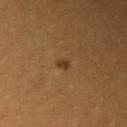Imaged during a routine full-body skin examination; the lesion was not biopsied and no histopathology is available.
The recorded lesion diameter is about 2 mm.
A 15 mm close-up extracted from a 3D total-body photography capture.
A female patient aged around 30.
Captured under cross-polarized illumination.
An algorithmic analysis of the crop reported a mean CIELAB color near L≈34 a*≈17 b*≈32, roughly 7 lightness units darker than nearby skin, and a lesion-to-skin contrast of about 7 (normalized; higher = more distinct).
The lesion is on the right upper arm.